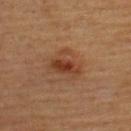  biopsy_status: not biopsied; imaged during a skin examination
  patient:
    sex: male
    age_approx: 60
  image:
    source: total-body photography crop
    field_of_view_mm: 15
  lesion_size:
    long_diameter_mm_approx: 4.0
  site: upper back
  lighting: cross-polarized